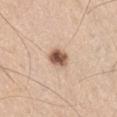{"biopsy_status": "not biopsied; imaged during a skin examination", "patient": {"sex": "male", "age_approx": 70}, "site": "abdomen", "image": {"source": "total-body photography crop", "field_of_view_mm": 15}, "lesion_size": {"long_diameter_mm_approx": 3.0}, "lighting": "white-light"}